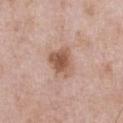The lesion was tiled from a total-body skin photograph and was not biopsied. A male patient roughly 50 years of age. About 4 mm across. From the chest. A lesion tile, about 15 mm wide, cut from a 3D total-body photograph. This is a white-light tile.